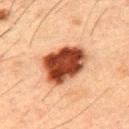Context:
A 15 mm crop from a total-body photograph taken for skin-cancer surveillance. A male subject in their 50s. Automated tile analysis of the lesion measured a footprint of about 20 mm², a shape eccentricity near 0.7, and a shape-asymmetry score of about 0.2 (0 = symmetric). The analysis additionally found a mean CIELAB color near L≈38 a*≈24 b*≈30, a lesion–skin lightness drop of about 20, and a lesion-to-skin contrast of about 15.5 (normalized; higher = more distinct). And it measured a border-irregularity rating of about 2/10, internal color variation of about 7 on a 0–10 scale, and peripheral color asymmetry of about 2. The analysis additionally found an automated nevus-likeness rating near 100 out of 100. Imaged with cross-polarized lighting. From the mid back.